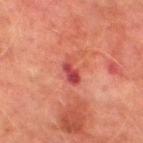Q: Was this lesion biopsied?
A: imaged on a skin check; not biopsied